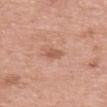Assessment:
The lesion was tiled from a total-body skin photograph and was not biopsied.
Image and clinical context:
From the upper back. A close-up tile cropped from a whole-body skin photograph, about 15 mm across. Captured under white-light illumination. A female subject aged around 70. The lesion-visualizer software estimated an outline eccentricity of about 0.85 (0 = round, 1 = elongated) and two-axis asymmetry of about 0.15.